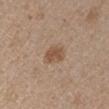Q: Was this lesion biopsied?
A: no biopsy performed (imaged during a skin exam)
Q: How large is the lesion?
A: ≈2.5 mm
Q: Where on the body is the lesion?
A: the arm
Q: What did automated image analysis measure?
A: a lesion area of about 5.5 mm² and a shape eccentricity near 0.55; a border-irregularity rating of about 2.5/10, internal color variation of about 2.5 on a 0–10 scale, and peripheral color asymmetry of about 1; an automated nevus-likeness rating near 65 out of 100 and lesion-presence confidence of about 100/100
Q: What is the imaging modality?
A: ~15 mm crop, total-body skin-cancer survey
Q: Who is the patient?
A: male, about 55 years old